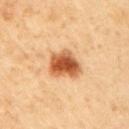Clinical impression: No biopsy was performed on this lesion — it was imaged during a full skin examination and was not determined to be concerning.